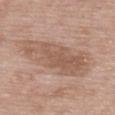No biopsy was performed on this lesion — it was imaged during a full skin examination and was not determined to be concerning.
Cropped from a total-body skin-imaging series; the visible field is about 15 mm.
The patient is a male aged approximately 85 or older.
On the upper back.